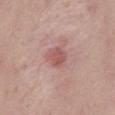{"biopsy_status": "not biopsied; imaged during a skin examination", "image": {"source": "total-body photography crop", "field_of_view_mm": 15}, "patient": {"sex": "male", "age_approx": 40}, "automated_metrics": {"cielab_L": 55, "cielab_a": 25, "cielab_b": 23, "vs_skin_darker_L": 9.0, "vs_skin_contrast_norm": 6.5, "border_irregularity_0_10": 1.5, "color_variation_0_10": 2.5, "peripheral_color_asymmetry": 1.0, "nevus_likeness_0_100": 25, "lesion_detection_confidence_0_100": 100}, "lesion_size": {"long_diameter_mm_approx": 2.5}, "site": "mid back", "lighting": "white-light"}